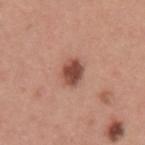{"site": "left upper arm", "lighting": "white-light", "image": {"source": "total-body photography crop", "field_of_view_mm": 15}, "patient": {"sex": "male", "age_approx": 40}}